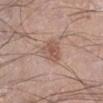Recorded during total-body skin imaging; not selected for excision or biopsy.
Located on the left lower leg.
The recorded lesion diameter is about 3 mm.
Captured under white-light illumination.
A male patient about 50 years old.
A roughly 15 mm field-of-view crop from a total-body skin photograph.